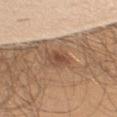Recorded during total-body skin imaging; not selected for excision or biopsy. A 15 mm close-up extracted from a 3D total-body photography capture. The tile uses white-light illumination. The lesion's longest dimension is about 3.5 mm. A male patient about 50 years old. Located on the right upper arm.